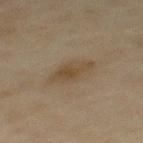• imaging modality — 15 mm crop, total-body photography
• lighting — cross-polarized
• image-analysis metrics — a footprint of about 8.5 mm², an eccentricity of roughly 0.85, and a shape-asymmetry score of about 0.15 (0 = symmetric); a border-irregularity rating of about 2.5/10, a color-variation rating of about 2.5/10, and peripheral color asymmetry of about 0.5; a nevus-likeness score of about 25/100 and a detector confidence of about 100 out of 100 that the crop contains a lesion
• location — the mid back
• patient — male, aged around 45
• diameter — ≈4.5 mm